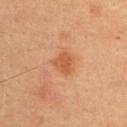follow-up = imaged on a skin check; not biopsied
lighting = cross-polarized
subject = female, aged approximately 30
image = ~15 mm tile from a whole-body skin photo
site = the back